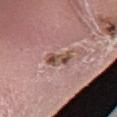Impression:
Imaged during a routine full-body skin examination; the lesion was not biopsied and no histopathology is available.
Background:
A lesion tile, about 15 mm wide, cut from a 3D total-body photograph. This is a white-light tile. The lesion is located on the leg. Automated image analysis of the tile measured an area of roughly 5 mm² and two-axis asymmetry of about 0.25. The software also gave about 12 CIELAB-L* units darker than the surrounding skin and a normalized lesion–skin contrast near 8.5. The software also gave a classifier nevus-likeness of about 10/100 and lesion-presence confidence of about 100/100. A male subject aged 53 to 57.